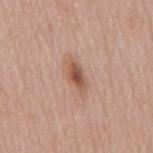Case summary:
– follow-up — catalogued during a skin exam; not biopsied
– image source — ~15 mm tile from a whole-body skin photo
– size — ~4 mm (longest diameter)
– body site — the mid back
– patient — male, in their 80s
– lighting — white-light illumination
– automated metrics — an average lesion color of about L≈54 a*≈21 b*≈29 (CIELAB), roughly 12 lightness units darker than nearby skin, and a normalized lesion–skin contrast near 8.5; a detector confidence of about 100 out of 100 that the crop contains a lesion